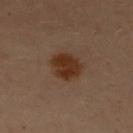Captured during whole-body skin photography for melanoma surveillance; the lesion was not biopsied. Imaged with cross-polarized lighting. The total-body-photography lesion software estimated an area of roughly 9 mm² and a shape eccentricity near 0.5. The software also gave border irregularity of about 2 on a 0–10 scale and a within-lesion color-variation index near 2/10. And it measured a nevus-likeness score of about 100/100. Cropped from a whole-body photographic skin survey; the tile spans about 15 mm. A female subject, aged approximately 50. Located on the chest. Approximately 3.5 mm at its widest.